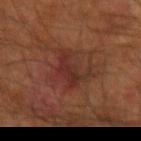Assessment:
The lesion was tiled from a total-body skin photograph and was not biopsied.
Background:
A close-up tile cropped from a whole-body skin photograph, about 15 mm across. Automated tile analysis of the lesion measured a shape eccentricity near 0.65 and two-axis asymmetry of about 0.45. It also reported peripheral color asymmetry of about 1.5. And it measured a lesion-detection confidence of about 100/100. Longest diameter approximately 5 mm. This is a cross-polarized tile. A male subject, aged around 60. On the right forearm.